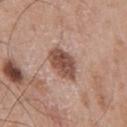Notes:
* biopsy status — no biopsy performed (imaged during a skin exam)
* image-analysis metrics — an area of roughly 9.5 mm², an outline eccentricity of about 0.75 (0 = round, 1 = elongated), and a symmetry-axis asymmetry near 0.15; a mean CIELAB color near L≈50 a*≈21 b*≈26
* patient — male, in their mid-60s
* site — the chest
* size — about 4.5 mm
* tile lighting — white-light
* image source — 15 mm crop, total-body photography